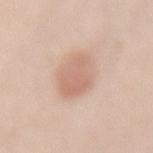workup: total-body-photography surveillance lesion; no biopsy
subject: female, approximately 40 years of age
site: the lower back
image: 15 mm crop, total-body photography